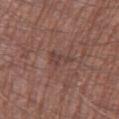Context:
Cropped from a whole-body photographic skin survey; the tile spans about 15 mm. Longest diameter approximately 3.5 mm. On the leg. The total-body-photography lesion software estimated an outline eccentricity of about 0.8 (0 = round, 1 = elongated) and two-axis asymmetry of about 0.4. A male subject in their mid- to late 60s. The tile uses white-light illumination.